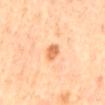biopsy status=total-body-photography surveillance lesion; no biopsy
diameter=~3 mm (longest diameter)
TBP lesion metrics=a footprint of about 3.5 mm², a shape eccentricity near 0.85, and a symmetry-axis asymmetry near 0.2; about 13 CIELAB-L* units darker than the surrounding skin; internal color variation of about 3.5 on a 0–10 scale and peripheral color asymmetry of about 1; a classifier nevus-likeness of about 40/100 and lesion-presence confidence of about 100/100
tile lighting=cross-polarized illumination
patient=female, aged 63 to 67
anatomic site=the mid back
imaging modality=~15 mm crop, total-body skin-cancer survey